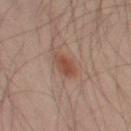Assessment:
Recorded during total-body skin imaging; not selected for excision or biopsy.
Acquisition and patient details:
The recorded lesion diameter is about 3 mm. A 15 mm close-up tile from a total-body photography series done for melanoma screening. On the left thigh. A male subject approximately 40 years of age. Imaged with cross-polarized lighting. Automated tile analysis of the lesion measured an area of roughly 5 mm², a shape eccentricity near 0.8, and two-axis asymmetry of about 0.2. The analysis additionally found a border-irregularity rating of about 2/10, a color-variation rating of about 2/10, and a peripheral color-asymmetry measure near 1.